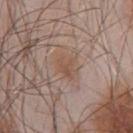This lesion was catalogued during total-body skin photography and was not selected for biopsy. About 3.5 mm across. The lesion is on the chest. This image is a 15 mm lesion crop taken from a total-body photograph. A male patient approximately 55 years of age. The tile uses white-light illumination. Automated tile analysis of the lesion measured a lesion color around L≈52 a*≈17 b*≈27 in CIELAB, roughly 6 lightness units darker than nearby skin, and a normalized border contrast of about 6. And it measured a within-lesion color-variation index near 3/10 and radial color variation of about 1.